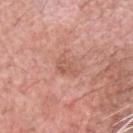Case summary:
- notes — total-body-photography surveillance lesion; no biopsy
- image source — total-body-photography crop, ~15 mm field of view
- lesion size — ≈2.5 mm
- body site — the head or neck
- patient — male, about 60 years old